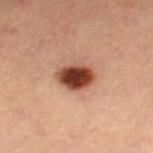<lesion>
<biopsy_status>not biopsied; imaged during a skin examination</biopsy_status>
<patient>
  <sex>female</sex>
  <age_approx>45</age_approx>
</patient>
<site>right thigh</site>
<image>
  <source>total-body photography crop</source>
  <field_of_view_mm>15</field_of_view_mm>
</image>
<automated_metrics>
  <area_mm2_approx>9.5</area_mm2_approx>
  <eccentricity>0.65</eccentricity>
  <shape_asymmetry>0.15</shape_asymmetry>
  <cielab_L>43</cielab_L>
  <cielab_a>25</cielab_a>
  <cielab_b>31</cielab_b>
  <vs_skin_darker_L>20.0</vs_skin_darker_L>
  <vs_skin_contrast_norm>14.0</vs_skin_contrast_norm>
  <nevus_likeness_0_100>100</nevus_likeness_0_100>
  <lesion_detection_confidence_0_100>100</lesion_detection_confidence_0_100>
</automated_metrics>
<lighting>cross-polarized</lighting>
<lesion_size>
  <long_diameter_mm_approx>4.0</long_diameter_mm_approx>
</lesion_size>
</lesion>